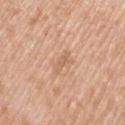Captured during whole-body skin photography for melanoma surveillance; the lesion was not biopsied. Imaged with white-light lighting. The recorded lesion diameter is about 3.5 mm. A close-up tile cropped from a whole-body skin photograph, about 15 mm across. Located on the left upper arm. The lesion-visualizer software estimated border irregularity of about 5 on a 0–10 scale and internal color variation of about 0 on a 0–10 scale. A male subject about 50 years old.